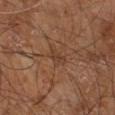This lesion was catalogued during total-body skin photography and was not selected for biopsy. A male subject aged 58–62. On the right leg. A roughly 15 mm field-of-view crop from a total-body skin photograph.